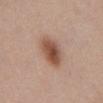| feature | finding |
|---|---|
| notes | total-body-photography surveillance lesion; no biopsy |
| image | ~15 mm tile from a whole-body skin photo |
| subject | female, aged 53–57 |
| anatomic site | the abdomen |
| illumination | white-light illumination |
| size | ≈4 mm |